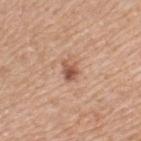This lesion was catalogued during total-body skin photography and was not selected for biopsy. An algorithmic analysis of the crop reported a footprint of about 4.5 mm² and an outline eccentricity of about 0.7 (0 = round, 1 = elongated). It also reported a classifier nevus-likeness of about 80/100 and a detector confidence of about 100 out of 100 that the crop contains a lesion. This is a white-light tile. A 15 mm close-up extracted from a 3D total-body photography capture. The lesion is on the upper back. A female subject, aged around 55.